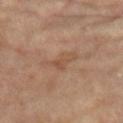{"biopsy_status": "not biopsied; imaged during a skin examination", "lighting": "cross-polarized", "site": "left lower leg", "lesion_size": {"long_diameter_mm_approx": 4.0}, "image": {"source": "total-body photography crop", "field_of_view_mm": 15}, "patient": {"sex": "female", "age_approx": 75}}